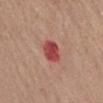{"biopsy_status": "not biopsied; imaged during a skin examination", "site": "front of the torso", "image": {"source": "total-body photography crop", "field_of_view_mm": 15}, "patient": {"sex": "female", "age_approx": 50}, "lesion_size": {"long_diameter_mm_approx": 3.0}, "lighting": "white-light"}